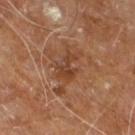{
  "biopsy_status": "not biopsied; imaged during a skin examination",
  "patient": {
    "sex": "male",
    "age_approx": 60
  },
  "lighting": "cross-polarized",
  "image": {
    "source": "total-body photography crop",
    "field_of_view_mm": 15
  },
  "site": "right leg"
}